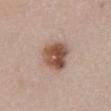follow-up — no biopsy performed (imaged during a skin exam) | location — the abdomen | patient — male, roughly 55 years of age | imaging modality — ~15 mm tile from a whole-body skin photo.